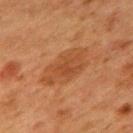image:
  source: total-body photography crop
  field_of_view_mm: 15
patient:
  sex: female
  age_approx: 40
lighting: cross-polarized
automated_metrics:
  eccentricity: 0.9
  cielab_L: 42
  cielab_a: 23
  cielab_b: 34
  border_irregularity_0_10: 2.5
  color_variation_0_10: 2.5
  peripheral_color_asymmetry: 0.5
  lesion_detection_confidence_0_100: 100
site: mid back
lesion_size:
  long_diameter_mm_approx: 6.5